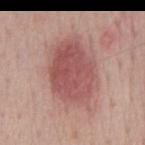biopsy status: catalogued during a skin exam; not biopsied
tile lighting: white-light
body site: the back
image source: total-body-photography crop, ~15 mm field of view
patient: male, in their mid- to late 50s
automated metrics: a normalized lesion–skin contrast near 8; a border-irregularity rating of about 2/10 and a color-variation rating of about 4/10; lesion-presence confidence of about 100/100
lesion diameter: ~7.5 mm (longest diameter)